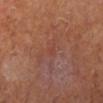biopsy_status: not biopsied; imaged during a skin examination
image:
  source: total-body photography crop
  field_of_view_mm: 15
lesion_size:
  long_diameter_mm_approx: 1.5
lighting: cross-polarized
patient:
  sex: female
site: right lower leg